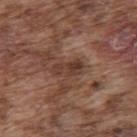The lesion was tiled from a total-body skin photograph and was not biopsied. The tile uses white-light illumination. A lesion tile, about 15 mm wide, cut from a 3D total-body photograph. About 3.5 mm across. The lesion is on the upper back. A male subject in their mid-70s.